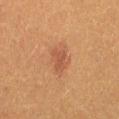Assessment: No biopsy was performed on this lesion — it was imaged during a full skin examination and was not determined to be concerning.